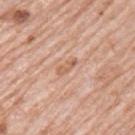Recorded during total-body skin imaging; not selected for excision or biopsy.
Automated image analysis of the tile measured a mean CIELAB color near L≈61 a*≈22 b*≈32, about 9 CIELAB-L* units darker than the surrounding skin, and a normalized lesion–skin contrast near 6. The software also gave an automated nevus-likeness rating near 0 out of 100 and a detector confidence of about 100 out of 100 that the crop contains a lesion.
The recorded lesion diameter is about 2.5 mm.
Cropped from a whole-body photographic skin survey; the tile spans about 15 mm.
A male patient, aged around 80.
The tile uses white-light illumination.
Located on the arm.